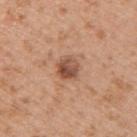<record>
<biopsy_status>not biopsied; imaged during a skin examination</biopsy_status>
<site>right upper arm</site>
<patient>
  <sex>male</sex>
  <age_approx>30</age_approx>
</patient>
<image>
  <source>total-body photography crop</source>
  <field_of_view_mm>15</field_of_view_mm>
</image>
</record>